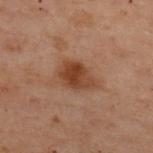Context: Automated image analysis of the tile measured about 9 CIELAB-L* units darker than the surrounding skin and a normalized lesion–skin contrast near 9. A female subject in their mid-50s. A 15 mm crop from a total-body photograph taken for skin-cancer surveillance. On the upper back. Captured under cross-polarized illumination.